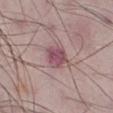From the right lower leg. The subject is a male about 50 years old. A 15 mm crop from a total-body photograph taken for skin-cancer surveillance.